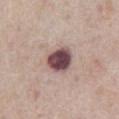Part of a total-body skin-imaging series; this lesion was reviewed on a skin check and was not flagged for biopsy.
The lesion's longest dimension is about 3.5 mm.
Automated tile analysis of the lesion measured a footprint of about 9 mm² and an eccentricity of roughly 0.45. The analysis additionally found a mean CIELAB color near L≈47 a*≈20 b*≈16 and a normalized lesion–skin contrast near 14. The analysis additionally found a border-irregularity index near 1.5/10 and radial color variation of about 1.5.
A lesion tile, about 15 mm wide, cut from a 3D total-body photograph.
A male subject, in their mid- to late 70s.
Captured under white-light illumination.
The lesion is located on the abdomen.